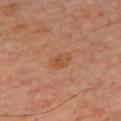<case>
  <biopsy_status>not biopsied; imaged during a skin examination</biopsy_status>
  <site>upper back</site>
  <patient>
    <sex>male</sex>
    <age_approx>60</age_approx>
  </patient>
  <image>
    <source>total-body photography crop</source>
    <field_of_view_mm>15</field_of_view_mm>
  </image>
  <lighting>cross-polarized</lighting>
</case>